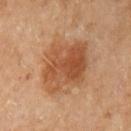Notes:
– notes: no biopsy performed (imaged during a skin exam)
– lesion diameter: ≈6 mm
– image-analysis metrics: a shape-asymmetry score of about 0.2 (0 = symmetric); a classifier nevus-likeness of about 60/100 and a lesion-detection confidence of about 100/100
– acquisition: ~15 mm tile from a whole-body skin photo
– subject: female, in their 60s
– body site: the left upper arm
– illumination: cross-polarized illumination